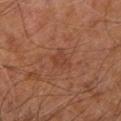{"biopsy_status": "not biopsied; imaged during a skin examination", "patient": {"sex": "male", "age_approx": 60}, "lighting": "cross-polarized", "image": {"source": "total-body photography crop", "field_of_view_mm": 15}, "lesion_size": {"long_diameter_mm_approx": 3.0}, "site": "right leg"}